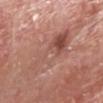The lesion is located on the head or neck.
About 10 mm across.
A close-up tile cropped from a whole-body skin photograph, about 15 mm across.
The patient is a male in their mid-60s.